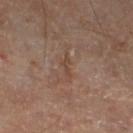Assessment: Part of a total-body skin-imaging series; this lesion was reviewed on a skin check and was not flagged for biopsy.